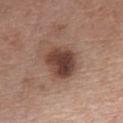Recorded during total-body skin imaging; not selected for excision or biopsy.
Captured under white-light illumination.
The subject is a female roughly 60 years of age.
The recorded lesion diameter is about 4.5 mm.
A roughly 15 mm field-of-view crop from a total-body skin photograph.
The lesion-visualizer software estimated an average lesion color of about L≈42 a*≈20 b*≈25 (CIELAB) and a normalized border contrast of about 10.5. And it measured a border-irregularity rating of about 1.5/10 and a within-lesion color-variation index near 6/10. The analysis additionally found a classifier nevus-likeness of about 80/100.
The lesion is on the chest.